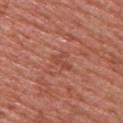workup: total-body-photography surveillance lesion; no biopsy | automated metrics: a lesion color around L≈48 a*≈28 b*≈31 in CIELAB and a lesion-to-skin contrast of about 5 (normalized; higher = more distinct) | site: the upper back | lesion size: about 3 mm | subject: female, about 55 years old | imaging modality: ~15 mm crop, total-body skin-cancer survey.